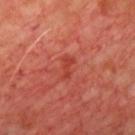| feature | finding |
|---|---|
| biopsy status | imaged on a skin check; not biopsied |
| illumination | cross-polarized illumination |
| automated metrics | border irregularity of about 4.5 on a 0–10 scale and radial color variation of about 0 |
| patient | male, in their 60s |
| location | the chest |
| image source | ~15 mm tile from a whole-body skin photo |